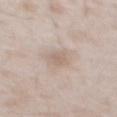biopsy status: no biopsy performed (imaged during a skin exam).